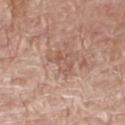This lesion was catalogued during total-body skin photography and was not selected for biopsy.
Automated tile analysis of the lesion measured a shape eccentricity near 0.75 and a shape-asymmetry score of about 0.45 (0 = symmetric). It also reported a border-irregularity rating of about 5.5/10, a within-lesion color-variation index near 2.5/10, and peripheral color asymmetry of about 1. It also reported a nevus-likeness score of about 0/100.
This image is a 15 mm lesion crop taken from a total-body photograph.
Measured at roughly 3.5 mm in maximum diameter.
The subject is a male aged approximately 70.
The tile uses white-light illumination.
On the right thigh.